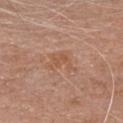{"patient": {"sex": "female", "age_approx": 75}, "image": {"source": "total-body photography crop", "field_of_view_mm": 15}, "site": "head or neck"}